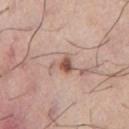No biopsy was performed on this lesion — it was imaged during a full skin examination and was not determined to be concerning. Approximately 3 mm at its widest. The tile uses white-light illumination. A close-up tile cropped from a whole-body skin photograph, about 15 mm across. The lesion is located on the chest. A male patient, aged approximately 75.